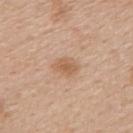{
  "biopsy_status": "not biopsied; imaged during a skin examination",
  "patient": {
    "sex": "male",
    "age_approx": 50
  },
  "lesion_size": {
    "long_diameter_mm_approx": 3.0
  },
  "image": {
    "source": "total-body photography crop",
    "field_of_view_mm": 15
  },
  "site": "mid back"
}